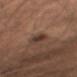Recorded during total-body skin imaging; not selected for excision or biopsy. The lesion is on the chest. A male subject aged 53–57. The tile uses white-light illumination. The lesion's longest dimension is about 3.5 mm. Automated tile analysis of the lesion measured a footprint of about 4.5 mm². The analysis additionally found a color-variation rating of about 4.5/10 and a peripheral color-asymmetry measure near 1.5. A region of skin cropped from a whole-body photographic capture, roughly 15 mm wide.